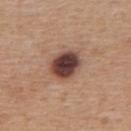Background:
A region of skin cropped from a whole-body photographic capture, roughly 15 mm wide. This is a white-light tile. The lesion's longest dimension is about 4 mm. The total-body-photography lesion software estimated an eccentricity of roughly 0.55 and two-axis asymmetry of about 0.15. And it measured an average lesion color of about L≈41 a*≈20 b*≈23 (CIELAB), a lesion–skin lightness drop of about 19, and a normalized border contrast of about 14.5. The software also gave a border-irregularity rating of about 1.5/10, a within-lesion color-variation index near 6/10, and radial color variation of about 1.5. The software also gave an automated nevus-likeness rating near 55 out of 100 and lesion-presence confidence of about 100/100. From the upper back. The patient is a female roughly 40 years of age.
Conclusion:
Biopsy histopathology demonstrated a benign lesion: dysplastic (Clark) nevus.